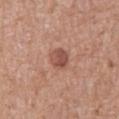Recorded during total-body skin imaging; not selected for excision or biopsy.
A 15 mm close-up extracted from a 3D total-body photography capture.
Captured under white-light illumination.
From the abdomen.
A male subject in their mid- to late 70s.
Automated image analysis of the tile measured border irregularity of about 1 on a 0–10 scale, internal color variation of about 4 on a 0–10 scale, and radial color variation of about 1.5.